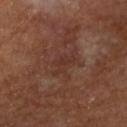Recorded during total-body skin imaging; not selected for excision or biopsy.